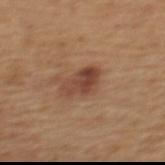Assessment: No biopsy was performed on this lesion — it was imaged during a full skin examination and was not determined to be concerning. Acquisition and patient details: A lesion tile, about 15 mm wide, cut from a 3D total-body photograph. On the back. The patient is a female aged approximately 55. An algorithmic analysis of the crop reported an area of roughly 8 mm² and two-axis asymmetry of about 0.2. And it measured a border-irregularity index near 2.5/10, a within-lesion color-variation index near 5.5/10, and peripheral color asymmetry of about 2. The software also gave a classifier nevus-likeness of about 90/100 and a detector confidence of about 100 out of 100 that the crop contains a lesion.